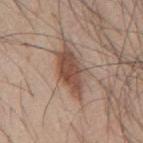Q: Was this lesion biopsied?
A: no biopsy performed (imaged during a skin exam)
Q: Who is the patient?
A: male, aged around 45
Q: What is the anatomic site?
A: the mid back
Q: What is the imaging modality?
A: total-body-photography crop, ~15 mm field of view